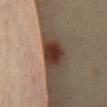The lesion was tiled from a total-body skin photograph and was not biopsied. A female patient, roughly 35 years of age. A region of skin cropped from a whole-body photographic capture, roughly 15 mm wide. An algorithmic analysis of the crop reported a footprint of about 9 mm², an eccentricity of roughly 0.85, and two-axis asymmetry of about 0.2. And it measured a border-irregularity index near 2/10, a within-lesion color-variation index near 5/10, and radial color variation of about 1.5. And it measured a classifier nevus-likeness of about 100/100 and lesion-presence confidence of about 100/100. The tile uses cross-polarized illumination. The lesion is located on the chest. Approximately 4.5 mm at its widest.